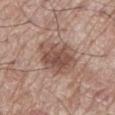Context: A male patient, aged approximately 70. Automated image analysis of the tile measured a border-irregularity index near 2/10, internal color variation of about 4.5 on a 0–10 scale, and a peripheral color-asymmetry measure near 1.5. Imaged with white-light lighting. On the leg. Cropped from a total-body skin-imaging series; the visible field is about 15 mm. About 5 mm across.